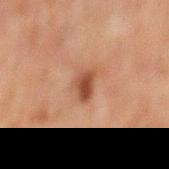Clinical impression: This lesion was catalogued during total-body skin photography and was not selected for biopsy. Context: The lesion-visualizer software estimated border irregularity of about 3 on a 0–10 scale, internal color variation of about 3 on a 0–10 scale, and radial color variation of about 1. The software also gave a classifier nevus-likeness of about 90/100 and lesion-presence confidence of about 100/100. A roughly 15 mm field-of-view crop from a total-body skin photograph. Longest diameter approximately 3 mm. The patient is a male aged around 60. The lesion is located on the mid back.